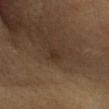* notes — total-body-photography surveillance lesion; no biopsy
* subject — female, aged approximately 45
* image source — 15 mm crop, total-body photography
* tile lighting — cross-polarized illumination
* lesion diameter — ~2.5 mm (longest diameter)
* body site — the head or neck